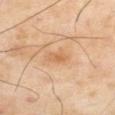Background: On the right thigh. A close-up tile cropped from a whole-body skin photograph, about 15 mm across. A male patient about 55 years old.